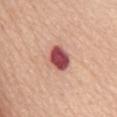| feature | finding |
|---|---|
| workup | imaged on a skin check; not biopsied |
| automated lesion analysis | a nevus-likeness score of about 10/100 and a detector confidence of about 100 out of 100 that the crop contains a lesion |
| imaging modality | ~15 mm crop, total-body skin-cancer survey |
| anatomic site | the back |
| subject | female, aged around 70 |
| lesion diameter | ≈3.5 mm |
| tile lighting | white-light |